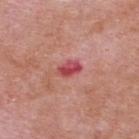Imaged during a routine full-body skin examination; the lesion was not biopsied and no histopathology is available. The lesion is located on the upper back. A male patient aged approximately 65. About 3 mm across. Cropped from a total-body skin-imaging series; the visible field is about 15 mm. The tile uses white-light illumination. An algorithmic analysis of the crop reported a footprint of about 4 mm², a shape eccentricity near 0.8, and a shape-asymmetry score of about 0.25 (0 = symmetric). The software also gave a mean CIELAB color near L≈50 a*≈38 b*≈24.